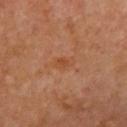Clinical impression:
The lesion was tiled from a total-body skin photograph and was not biopsied.
Context:
The total-body-photography lesion software estimated a shape eccentricity near 0.8 and two-axis asymmetry of about 0.25. The analysis additionally found a lesion color around L≈47 a*≈24 b*≈36 in CIELAB, about 5 CIELAB-L* units darker than the surrounding skin, and a normalized lesion–skin contrast near 5.5. The software also gave border irregularity of about 2.5 on a 0–10 scale, internal color variation of about 3 on a 0–10 scale, and peripheral color asymmetry of about 1. A female subject, aged around 65. The tile uses cross-polarized illumination. From the front of the torso. Cropped from a whole-body photographic skin survey; the tile spans about 15 mm. The lesion's longest dimension is about 2.5 mm.